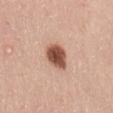Part of a total-body skin-imaging series; this lesion was reviewed on a skin check and was not flagged for biopsy. The tile uses white-light illumination. The lesion is located on the lower back. A female subject, aged approximately 45. This image is a 15 mm lesion crop taken from a total-body photograph.